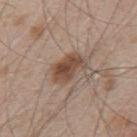Longest diameter approximately 4 mm. A male subject, aged around 50. From the mid back. A 15 mm close-up tile from a total-body photography series done for melanoma screening.